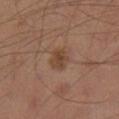The lesion was photographed on a routine skin check and not biopsied; there is no pathology result. On the right lower leg. The lesion-visualizer software estimated a footprint of about 5 mm², an eccentricity of roughly 0.45, and a shape-asymmetry score of about 0.15 (0 = symmetric). And it measured an average lesion color of about L≈42 a*≈17 b*≈28 (CIELAB). This is a cross-polarized tile. A male subject aged 43 to 47. Longest diameter approximately 2.5 mm. A close-up tile cropped from a whole-body skin photograph, about 15 mm across.